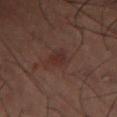workup — no biopsy performed (imaged during a skin exam); image source — ~15 mm crop, total-body skin-cancer survey; patient — female, about 55 years old; location — the leg; lesion size — ~2.5 mm (longest diameter); lighting — cross-polarized illumination.